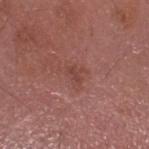This lesion was catalogued during total-body skin photography and was not selected for biopsy. The total-body-photography lesion software estimated a footprint of about 1.5 mm², an outline eccentricity of about 0.9 (0 = round, 1 = elongated), and a shape-asymmetry score of about 0.25 (0 = symmetric). And it measured a nevus-likeness score of about 0/100 and lesion-presence confidence of about 100/100. On the head or neck. This is a white-light tile. The recorded lesion diameter is about 2 mm. Cropped from a total-body skin-imaging series; the visible field is about 15 mm. The patient is a male aged around 55.